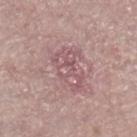<lesion>
  <lesion_size>
    <long_diameter_mm_approx>4.5</long_diameter_mm_approx>
  </lesion_size>
  <patient>
    <sex>female</sex>
    <age_approx>70</age_approx>
  </patient>
  <site>leg</site>
  <image>
    <source>total-body photography crop</source>
    <field_of_view_mm>15</field_of_view_mm>
  </image>
</lesion>